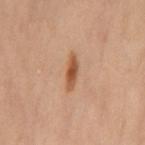biopsy status: catalogued during a skin exam; not biopsied | subject: female, aged 38–42 | imaging modality: total-body-photography crop, ~15 mm field of view | anatomic site: the right leg | lesion diameter: ~4 mm (longest diameter).